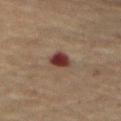Captured during whole-body skin photography for melanoma surveillance; the lesion was not biopsied. A 15 mm crop from a total-body photograph taken for skin-cancer surveillance. A male patient roughly 65 years of age. About 3 mm across. On the abdomen. Captured under cross-polarized illumination.